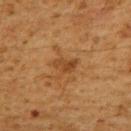Recorded during total-body skin imaging; not selected for excision or biopsy. A male subject, aged around 60. On the upper back. This image is a 15 mm lesion crop taken from a total-body photograph. Captured under cross-polarized illumination. About 2.5 mm across. An algorithmic analysis of the crop reported an area of roughly 4 mm², a shape eccentricity near 0.8, and a shape-asymmetry score of about 0.45 (0 = symmetric). The software also gave a border-irregularity rating of about 4/10, a within-lesion color-variation index near 2/10, and peripheral color asymmetry of about 0.5. And it measured a classifier nevus-likeness of about 5/100 and a detector confidence of about 100 out of 100 that the crop contains a lesion.